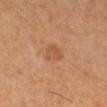The lesion was photographed on a routine skin check and not biopsied; there is no pathology result. A female patient, aged around 50. Cropped from a whole-body photographic skin survey; the tile spans about 15 mm. Longest diameter approximately 3 mm.